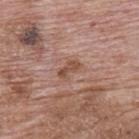The lesion was photographed on a routine skin check and not biopsied; there is no pathology result. This is a white-light tile. A male patient roughly 70 years of age. Cropped from a total-body skin-imaging series; the visible field is about 15 mm. On the upper back.